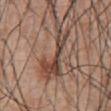From the front of the torso. A male subject, aged 53–57. The tile uses white-light illumination. Approximately 7.5 mm at its widest. A 15 mm close-up tile from a total-body photography series done for melanoma screening. An algorithmic analysis of the crop reported a footprint of about 13 mm².